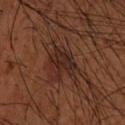Clinical impression:
The lesion was tiled from a total-body skin photograph and was not biopsied.
Clinical summary:
This image is a 15 mm lesion crop taken from a total-body photograph. From the upper back. An algorithmic analysis of the crop reported an area of roughly 8 mm² and an eccentricity of roughly 0.4. It also reported an average lesion color of about L≈24 a*≈18 b*≈22 (CIELAB), about 7 CIELAB-L* units darker than the surrounding skin, and a normalized border contrast of about 8.5. It also reported a border-irregularity rating of about 5/10, a within-lesion color-variation index near 2.5/10, and a peripheral color-asymmetry measure near 1. And it measured a nevus-likeness score of about 5/100 and lesion-presence confidence of about 100/100. The lesion's longest dimension is about 3.5 mm. The patient is a male aged approximately 50.